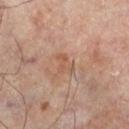{
  "biopsy_status": "not biopsied; imaged during a skin examination",
  "lighting": "cross-polarized",
  "patient": {
    "sex": "male",
    "age_approx": 65
  },
  "site": "left lower leg",
  "image": {
    "source": "total-body photography crop",
    "field_of_view_mm": 15
  },
  "lesion_size": {
    "long_diameter_mm_approx": 3.0
  }
}